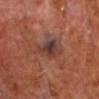This lesion was catalogued during total-body skin photography and was not selected for biopsy. Captured under cross-polarized illumination. The subject is a male aged 63 to 67. A 15 mm close-up tile from a total-body photography series done for melanoma screening. Measured at roughly 4 mm in maximum diameter. The lesion-visualizer software estimated a footprint of about 8 mm², an outline eccentricity of about 0.7 (0 = round, 1 = elongated), and two-axis asymmetry of about 0.35. It also reported a classifier nevus-likeness of about 0/100 and lesion-presence confidence of about 100/100. The lesion is located on the head or neck.